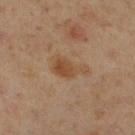Findings:
• notes · catalogued during a skin exam; not biopsied
• image source · ~15 mm crop, total-body skin-cancer survey
• illumination · cross-polarized illumination
• anatomic site · the right lower leg
• patient · male, aged 58 to 62